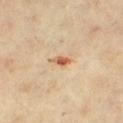notes = no biopsy performed (imaged during a skin exam)
patient = female, aged 63 to 67
diameter = ≈2.5 mm
location = the left lower leg
image-analysis metrics = a lesion area of about 2.5 mm²; roughly 14 lightness units darker than nearby skin and a normalized lesion–skin contrast near 9; a border-irregularity rating of about 2.5/10 and a color-variation rating of about 2/10; lesion-presence confidence of about 100/100
illumination = cross-polarized
imaging modality = 15 mm crop, total-body photography